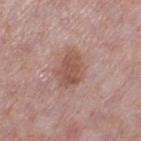The lesion was photographed on a routine skin check and not biopsied; there is no pathology result.
A female subject about 40 years old.
This image is a 15 mm lesion crop taken from a total-body photograph.
An algorithmic analysis of the crop reported a lesion area of about 10 mm² and a shape-asymmetry score of about 0.25 (0 = symmetric). The analysis additionally found a nevus-likeness score of about 10/100.
The lesion is on the left thigh.
Captured under white-light illumination.